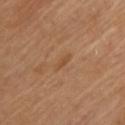Findings:
– follow-up: imaged on a skin check; not biopsied
– body site: the chest
– image source: 15 mm crop, total-body photography